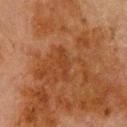Part of a total-body skin-imaging series; this lesion was reviewed on a skin check and was not flagged for biopsy. A male patient approximately 80 years of age. Imaged with cross-polarized lighting. Approximately 3.5 mm at its widest. Located on the back. Cropped from a whole-body photographic skin survey; the tile spans about 15 mm. Automated tile analysis of the lesion measured a lesion area of about 3.5 mm², an outline eccentricity of about 0.95 (0 = round, 1 = elongated), and a shape-asymmetry score of about 0.35 (0 = symmetric). It also reported a mean CIELAB color near L≈32 a*≈21 b*≈31, about 5 CIELAB-L* units darker than the surrounding skin, and a normalized lesion–skin contrast near 5.